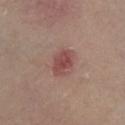biopsy status = imaged on a skin check; not biopsied | subject = female, aged approximately 55 | anatomic site = the left lower leg | tile lighting = cross-polarized | image source = ~15 mm tile from a whole-body skin photo.